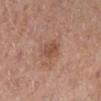No biopsy was performed on this lesion — it was imaged during a full skin examination and was not determined to be concerning. The lesion is on the left lower leg. An algorithmic analysis of the crop reported an area of roughly 5 mm², an outline eccentricity of about 0.7 (0 = round, 1 = elongated), and a shape-asymmetry score of about 0.15 (0 = symmetric). And it measured a mean CIELAB color near L≈50 a*≈22 b*≈29, a lesion–skin lightness drop of about 8, and a normalized border contrast of about 6. It also reported border irregularity of about 2 on a 0–10 scale, internal color variation of about 2 on a 0–10 scale, and radial color variation of about 0.5. A roughly 15 mm field-of-view crop from a total-body skin photograph. The patient is a female aged around 40.